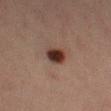patient: female, approximately 40 years of age | imaging modality: 15 mm crop, total-body photography | body site: the left thigh.